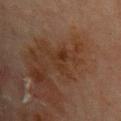Impression:
Imaged during a routine full-body skin examination; the lesion was not biopsied and no histopathology is available.
Acquisition and patient details:
A 15 mm close-up extracted from a 3D total-body photography capture. The lesion-visualizer software estimated a lesion area of about 5 mm² and a shape eccentricity near 0.75. The lesion is located on the chest. A male subject, aged approximately 70.